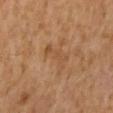Assessment:
Imaged during a routine full-body skin examination; the lesion was not biopsied and no histopathology is available.
Acquisition and patient details:
A lesion tile, about 15 mm wide, cut from a 3D total-body photograph. The patient is a male aged around 60. Measured at roughly 4.5 mm in maximum diameter. The lesion is on the mid back. The tile uses cross-polarized illumination.